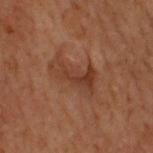biopsy status=total-body-photography surveillance lesion; no biopsy
acquisition=15 mm crop, total-body photography
anatomic site=the upper back
subject=male, aged 68 to 72
lighting=cross-polarized